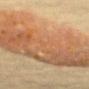Assessment:
Recorded during total-body skin imaging; not selected for excision or biopsy.
Image and clinical context:
A region of skin cropped from a whole-body photographic capture, roughly 15 mm wide. The lesion is located on the back. Approximately 17.5 mm at its widest. A female patient, roughly 80 years of age.